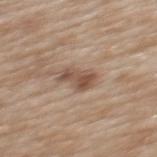| field | value |
|---|---|
| notes | catalogued during a skin exam; not biopsied |